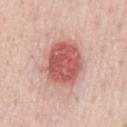notes = no biopsy performed (imaged during a skin exam); imaging modality = total-body-photography crop, ~15 mm field of view; body site = the chest; image-analysis metrics = an area of roughly 20 mm², a shape eccentricity near 0.6, and a symmetry-axis asymmetry near 0.15; patient = male, aged 48–52; size = about 5.5 mm.